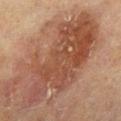{"biopsy_status": "not biopsied; imaged during a skin examination", "image": {"source": "total-body photography crop", "field_of_view_mm": 15}, "patient": {"sex": "female", "age_approx": 80}, "automated_metrics": {"area_mm2_approx": 60.0, "shape_asymmetry": 0.25, "border_irregularity_0_10": 6.0, "color_variation_0_10": 5.5, "peripheral_color_asymmetry": 1.5, "nevus_likeness_0_100": 95}, "lesion_size": {"long_diameter_mm_approx": 14.5}, "site": "left lower leg"}